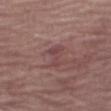A close-up tile cropped from a whole-body skin photograph, about 15 mm across. The lesion is on the right thigh. Imaged with white-light lighting. Approximately 3 mm at its widest. The subject is a female aged 83 or over.